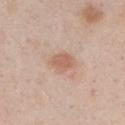Case summary:
• lighting — white-light illumination
• patient — male, aged around 50
• image source — total-body-photography crop, ~15 mm field of view
• diameter — ≈3.5 mm
• anatomic site — the chest